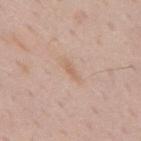Clinical impression: The lesion was tiled from a total-body skin photograph and was not biopsied. Context: A 15 mm close-up tile from a total-body photography series done for melanoma screening. A male patient approximately 70 years of age. Longest diameter approximately 3 mm. Automated tile analysis of the lesion measured an area of roughly 2.5 mm², an outline eccentricity of about 0.95 (0 = round, 1 = elongated), and a symmetry-axis asymmetry near 0.35. The analysis additionally found an average lesion color of about L≈63 a*≈18 b*≈30 (CIELAB) and roughly 6 lightness units darker than nearby skin. The software also gave border irregularity of about 4 on a 0–10 scale, a color-variation rating of about 0/10, and peripheral color asymmetry of about 0. And it measured an automated nevus-likeness rating near 0 out of 100 and lesion-presence confidence of about 100/100. The lesion is on the mid back.